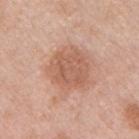{
  "biopsy_status": "not biopsied; imaged during a skin examination",
  "image": {
    "source": "total-body photography crop",
    "field_of_view_mm": 15
  },
  "patient": {
    "sex": "male",
    "age_approx": 45
  },
  "lesion_size": {
    "long_diameter_mm_approx": 5.0
  },
  "automated_metrics": {
    "area_mm2_approx": 16.0,
    "eccentricity": 0.4,
    "shape_asymmetry": 0.2,
    "cielab_L": 59,
    "cielab_a": 22,
    "cielab_b": 31,
    "vs_skin_darker_L": 9.0,
    "vs_skin_contrast_norm": 6.5,
    "peripheral_color_asymmetry": 1.0,
    "nevus_likeness_0_100": 25,
    "lesion_detection_confidence_0_100": 100
  },
  "lighting": "white-light",
  "site": "chest"
}